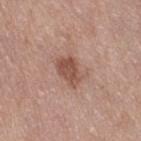Part of a total-body skin-imaging series; this lesion was reviewed on a skin check and was not flagged for biopsy.
A roughly 15 mm field-of-view crop from a total-body skin photograph.
Located on the right thigh.
The lesion's longest dimension is about 4 mm.
The subject is a female aged around 40.